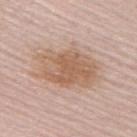| key | value |
|---|---|
| follow-up | imaged on a skin check; not biopsied |
| location | the upper back |
| lighting | white-light |
| TBP lesion metrics | a lesion area of about 24 mm², a shape eccentricity near 0.8, and a shape-asymmetry score of about 0.15 (0 = symmetric); a border-irregularity index near 3/10, a color-variation rating of about 4/10, and peripheral color asymmetry of about 1; an automated nevus-likeness rating near 20 out of 100 and a detector confidence of about 100 out of 100 that the crop contains a lesion |
| lesion size | ~7 mm (longest diameter) |
| patient | male, aged approximately 65 |
| acquisition | 15 mm crop, total-body photography |